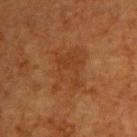Part of a total-body skin-imaging series; this lesion was reviewed on a skin check and was not flagged for biopsy. This image is a 15 mm lesion crop taken from a total-body photograph. The lesion is located on the upper back. A female subject in their 40s. Approximately 6 mm at its widest.